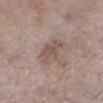biopsy status: total-body-photography surveillance lesion; no biopsy
site: the left lower leg
size: ~5 mm (longest diameter)
automated lesion analysis: a symmetry-axis asymmetry near 0.3; an average lesion color of about L≈53 a*≈15 b*≈22 (CIELAB), roughly 8 lightness units darker than nearby skin, and a normalized lesion–skin contrast near 6; a within-lesion color-variation index near 2/10 and radial color variation of about 0.5; a nevus-likeness score of about 5/100
tile lighting: white-light illumination
acquisition: ~15 mm tile from a whole-body skin photo
subject: female, aged 73 to 77